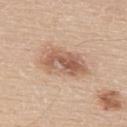The lesion was tiled from a total-body skin photograph and was not biopsied. Located on the back. An algorithmic analysis of the crop reported a color-variation rating of about 7/10. Cropped from a total-body skin-imaging series; the visible field is about 15 mm. This is a white-light tile. About 6 mm across. A male patient about 60 years old.